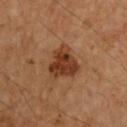A 15 mm crop from a total-body photograph taken for skin-cancer surveillance. The lesion is located on the arm. A male subject aged 53 to 57.